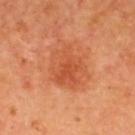Impression: Recorded during total-body skin imaging; not selected for excision or biopsy. Clinical summary: The recorded lesion diameter is about 5.5 mm. The subject is a male aged 63–67. On the upper back. A 15 mm close-up extracted from a 3D total-body photography capture. This is a cross-polarized tile.